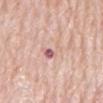Assessment:
Captured during whole-body skin photography for melanoma surveillance; the lesion was not biopsied.
Image and clinical context:
A male patient, aged around 80. A 15 mm close-up tile from a total-body photography series done for melanoma screening. The lesion is located on the mid back.